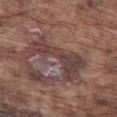  biopsy_status: not biopsied; imaged during a skin examination